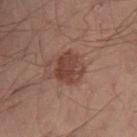Findings:
• notes: total-body-photography surveillance lesion; no biopsy
• tile lighting: cross-polarized illumination
• subject: male, roughly 50 years of age
• size: ~3.5 mm (longest diameter)
• image: 15 mm crop, total-body photography
• site: the left leg
• TBP lesion metrics: an area of roughly 9.5 mm², an eccentricity of roughly 0.5, and two-axis asymmetry of about 0.2; an automated nevus-likeness rating near 40 out of 100 and a detector confidence of about 100 out of 100 that the crop contains a lesion